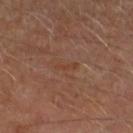Imaged during a routine full-body skin examination; the lesion was not biopsied and no histopathology is available. Imaged with cross-polarized lighting. The patient is a male aged 63 to 67. An algorithmic analysis of the crop reported an area of roughly 2 mm² and a shape-asymmetry score of about 0.4 (0 = symmetric). The software also gave a border-irregularity index near 5/10 and peripheral color asymmetry of about 0. A roughly 15 mm field-of-view crop from a total-body skin photograph. Approximately 2.5 mm at its widest. Located on the head or neck.